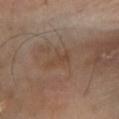| feature | finding |
|---|---|
| anatomic site | the leg |
| subject | male, aged 68–72 |
| automated metrics | a footprint of about 4.5 mm², an outline eccentricity of about 0.85 (0 = round, 1 = elongated), and a symmetry-axis asymmetry near 0.3; a lesion color around L≈42 a*≈16 b*≈27 in CIELAB, a lesion–skin lightness drop of about 5, and a normalized border contrast of about 5; a detector confidence of about 100 out of 100 that the crop contains a lesion |
| image source | 15 mm crop, total-body photography |